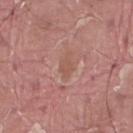notes=total-body-photography surveillance lesion; no biopsy
acquisition=15 mm crop, total-body photography
patient=male, aged approximately 40
body site=the right thigh
lesion size=~3 mm (longest diameter)
lighting=white-light